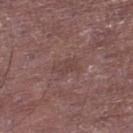workup: total-body-photography surveillance lesion; no biopsy
TBP lesion metrics: a footprint of about 4 mm², an eccentricity of roughly 0.85, and two-axis asymmetry of about 0.25; roughly 5 lightness units darker than nearby skin and a normalized border contrast of about 4.5; a border-irregularity rating of about 2.5/10, a within-lesion color-variation index near 1.5/10, and a peripheral color-asymmetry measure near 0.5; a classifier nevus-likeness of about 0/100 and a detector confidence of about 100 out of 100 that the crop contains a lesion
patient: male, aged 63 to 67
lighting: white-light
acquisition: ~15 mm tile from a whole-body skin photo
site: the left lower leg